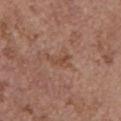| feature | finding |
|---|---|
| notes | total-body-photography surveillance lesion; no biopsy |
| body site | the chest |
| acquisition | ~15 mm tile from a whole-body skin photo |
| tile lighting | white-light illumination |
| patient | female, aged around 65 |